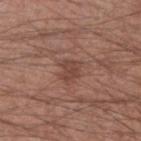Q: Is there a histopathology result?
A: imaged on a skin check; not biopsied
Q: Illumination type?
A: white-light illumination
Q: Patient demographics?
A: male, aged 53 to 57
Q: Lesion location?
A: the right forearm
Q: Automated lesion metrics?
A: a lesion area of about 5 mm², a shape eccentricity near 0.55, and a shape-asymmetry score of about 0.25 (0 = symmetric); an average lesion color of about L≈44 a*≈21 b*≈26 (CIELAB), a lesion–skin lightness drop of about 7, and a normalized border contrast of about 6; a border-irregularity index near 2.5/10, internal color variation of about 2.5 on a 0–10 scale, and radial color variation of about 1
Q: How was this image acquired?
A: total-body-photography crop, ~15 mm field of view
Q: What is the lesion's diameter?
A: about 2.5 mm